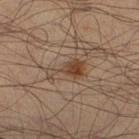The lesion is located on the left leg. Longest diameter approximately 4.5 mm. Captured under cross-polarized illumination. A male patient aged around 50. A roughly 15 mm field-of-view crop from a total-body skin photograph.